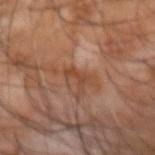The lesion was photographed on a routine skin check and not biopsied; there is no pathology result. The lesion's longest dimension is about 2.5 mm. From the arm. Captured under cross-polarized illumination. The lesion-visualizer software estimated a lesion area of about 2.5 mm², an outline eccentricity of about 0.85 (0 = round, 1 = elongated), and two-axis asymmetry of about 0.55. And it measured a mean CIELAB color near L≈41 a*≈21 b*≈29, a lesion–skin lightness drop of about 7, and a lesion-to-skin contrast of about 6.5 (normalized; higher = more distinct). It also reported a lesion-detection confidence of about 100/100. Cropped from a whole-body photographic skin survey; the tile spans about 15 mm. The subject is a male in their mid-50s.